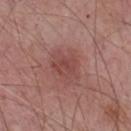notes = no biopsy performed (imaged during a skin exam).